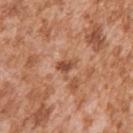The lesion was photographed on a routine skin check and not biopsied; there is no pathology result.
Located on the right upper arm.
This image is a 15 mm lesion crop taken from a total-body photograph.
The total-body-photography lesion software estimated a lesion area of about 4 mm², an eccentricity of roughly 0.7, and two-axis asymmetry of about 0.5. It also reported a peripheral color-asymmetry measure near 0.5.
The tile uses white-light illumination.
A male patient aged approximately 45.